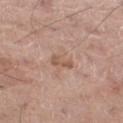Impression: Part of a total-body skin-imaging series; this lesion was reviewed on a skin check and was not flagged for biopsy. Acquisition and patient details: The recorded lesion diameter is about 2.5 mm. Captured under white-light illumination. A male patient, aged approximately 70. An algorithmic analysis of the crop reported an area of roughly 3.5 mm², a shape eccentricity near 0.8, and a symmetry-axis asymmetry near 0.25. And it measured a nevus-likeness score of about 0/100 and a detector confidence of about 100 out of 100 that the crop contains a lesion. Cropped from a total-body skin-imaging series; the visible field is about 15 mm. Located on the left thigh.